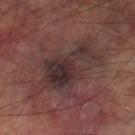Recorded during total-body skin imaging; not selected for excision or biopsy.
Located on the left thigh.
A lesion tile, about 15 mm wide, cut from a 3D total-body photograph.
The lesion-visualizer software estimated a lesion color around L≈29 a*≈16 b*≈15 in CIELAB, a lesion–skin lightness drop of about 8, and a lesion-to-skin contrast of about 9 (normalized; higher = more distinct). The software also gave a classifier nevus-likeness of about 0/100.
A male subject, aged around 70.
About 7.5 mm across.